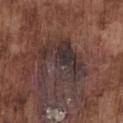Imaged during a routine full-body skin examination; the lesion was not biopsied and no histopathology is available. Longest diameter approximately 6 mm. A 15 mm close-up extracted from a 3D total-body photography capture. A male subject, aged 73 to 77. Located on the chest.